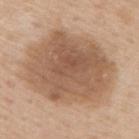<lesion>
  <biopsy_status>not biopsied; imaged during a skin examination</biopsy_status>
  <patient>
    <sex>male</sex>
    <age_approx>60</age_approx>
  </patient>
  <site>upper back</site>
  <lesion_size>
    <long_diameter_mm_approx>10.0</long_diameter_mm_approx>
  </lesion_size>
  <automated_metrics>
    <border_irregularity_0_10>1.5</border_irregularity_0_10>
    <peripheral_color_asymmetry>1.5</peripheral_color_asymmetry>
    <nevus_likeness_0_100>20</nevus_likeness_0_100>
    <lesion_detection_confidence_0_100>100</lesion_detection_confidence_0_100>
  </automated_metrics>
  <lighting>white-light</lighting>
  <image>
    <source>total-body photography crop</source>
    <field_of_view_mm>15</field_of_view_mm>
  </image>
</lesion>